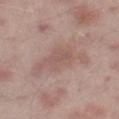Longest diameter approximately 7.5 mm. The patient is a male in their mid-60s. From the right thigh. Captured under white-light illumination. A roughly 15 mm field-of-view crop from a total-body skin photograph.